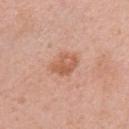<lesion>
<biopsy_status>not biopsied; imaged during a skin examination</biopsy_status>
<patient>
  <sex>female</sex>
  <age_approx>35</age_approx>
</patient>
<lesion_size>
  <long_diameter_mm_approx>3.5</long_diameter_mm_approx>
</lesion_size>
<site>arm</site>
<image>
  <source>total-body photography crop</source>
  <field_of_view_mm>15</field_of_view_mm>
</image>
<lighting>white-light</lighting>
</lesion>